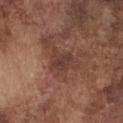This lesion was catalogued during total-body skin photography and was not selected for biopsy.
From the chest.
A 15 mm close-up tile from a total-body photography series done for melanoma screening.
A male patient aged 73 to 77.